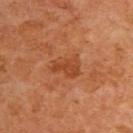No biopsy was performed on this lesion — it was imaged during a full skin examination and was not determined to be concerning.
Captured under cross-polarized illumination.
Cropped from a total-body skin-imaging series; the visible field is about 15 mm.
The recorded lesion diameter is about 3.5 mm.
The lesion is located on the upper back.
A male subject roughly 60 years of age.